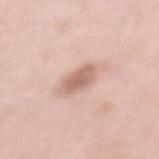Clinical impression: The lesion was photographed on a routine skin check and not biopsied; there is no pathology result. Acquisition and patient details: A close-up tile cropped from a whole-body skin photograph, about 15 mm across. The lesion is located on the mid back. A female patient aged 38 to 42.